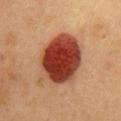<tbp_lesion>
<biopsy_status>not biopsied; imaged during a skin examination</biopsy_status>
<lighting>cross-polarized</lighting>
<image>
  <source>total-body photography crop</source>
  <field_of_view_mm>15</field_of_view_mm>
</image>
<patient>
  <sex>female</sex>
  <age_approx>40</age_approx>
</patient>
<site>chest</site>
</tbp_lesion>